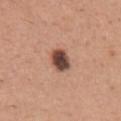| feature | finding |
|---|---|
| biopsy status | catalogued during a skin exam; not biopsied |
| patient | male, aged approximately 45 |
| tile lighting | white-light illumination |
| imaging modality | ~15 mm crop, total-body skin-cancer survey |
| location | the chest |